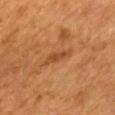follow-up: total-body-photography surveillance lesion; no biopsy | illumination: cross-polarized | anatomic site: the mid back | patient: female, approximately 55 years of age | automated lesion analysis: a lesion area of about 4 mm², a shape eccentricity near 0.95, and a symmetry-axis asymmetry near 0.35; an average lesion color of about L≈39 a*≈21 b*≈33 (CIELAB), about 7 CIELAB-L* units darker than the surrounding skin, and a lesion-to-skin contrast of about 6 (normalized; higher = more distinct); a classifier nevus-likeness of about 0/100 and lesion-presence confidence of about 85/100 | imaging modality: total-body-photography crop, ~15 mm field of view.